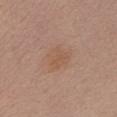Findings:
• workup · total-body-photography surveillance lesion; no biopsy